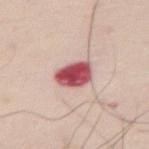tile lighting = white-light | imaging modality = ~15 mm tile from a whole-body skin photo | lesion size = ~4 mm (longest diameter) | anatomic site = the right thigh | subject = male, aged 58–62.